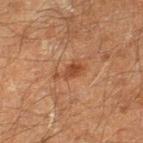Context:
A male subject aged around 60. Automated image analysis of the tile measured an average lesion color of about L≈36 a*≈20 b*≈28 (CIELAB), about 7 CIELAB-L* units darker than the surrounding skin, and a normalized lesion–skin contrast near 7. It also reported border irregularity of about 4.5 on a 0–10 scale and internal color variation of about 2.5 on a 0–10 scale. A 15 mm close-up extracted from a 3D total-body photography capture. This is a cross-polarized tile. Located on the left lower leg.